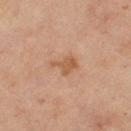Assessment: Imaged during a routine full-body skin examination; the lesion was not biopsied and no histopathology is available. Background: Measured at roughly 3 mm in maximum diameter. From the right thigh. Automated image analysis of the tile measured an average lesion color of about L≈48 a*≈19 b*≈31 (CIELAB), a lesion–skin lightness drop of about 7, and a normalized border contrast of about 6.5. The analysis additionally found a border-irregularity rating of about 4.5/10 and internal color variation of about 1.5 on a 0–10 scale. It also reported a classifier nevus-likeness of about 5/100 and lesion-presence confidence of about 100/100. A close-up tile cropped from a whole-body skin photograph, about 15 mm across. The patient is a female aged approximately 70.